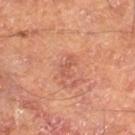follow-up — catalogued during a skin exam; not biopsied | imaging modality — ~15 mm crop, total-body skin-cancer survey | TBP lesion metrics — an area of roughly 3 mm², an outline eccentricity of about 0.9 (0 = round, 1 = elongated), and a symmetry-axis asymmetry near 0.4; border irregularity of about 4.5 on a 0–10 scale and a peripheral color-asymmetry measure near 0; a classifier nevus-likeness of about 0/100 | location — the right thigh | patient — male, aged 63 to 67.